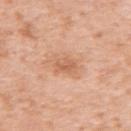The lesion was tiled from a total-body skin photograph and was not biopsied. The tile uses white-light illumination. Cropped from a total-body skin-imaging series; the visible field is about 15 mm. The subject is a female aged approximately 40. Located on the left upper arm.